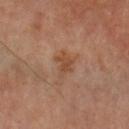Findings:
• illumination — cross-polarized
• location — the left forearm
• imaging modality — total-body-photography crop, ~15 mm field of view
• automated lesion analysis — a border-irregularity rating of about 4.5/10, a color-variation rating of about 1.5/10, and peripheral color asymmetry of about 0.5; an automated nevus-likeness rating near 0 out of 100 and a lesion-detection confidence of about 100/100
• patient — male, about 70 years old